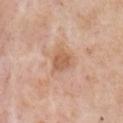Q: Was a biopsy performed?
A: total-body-photography surveillance lesion; no biopsy
Q: Patient demographics?
A: male, about 75 years old
Q: What kind of image is this?
A: ~15 mm tile from a whole-body skin photo
Q: Illumination type?
A: white-light illumination
Q: What is the anatomic site?
A: the right upper arm